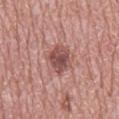• follow-up — total-body-photography surveillance lesion; no biopsy
• lighting — white-light
• patient — male, aged around 70
• imaging modality — ~15 mm crop, total-body skin-cancer survey
• site — the back